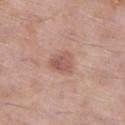– follow-up · catalogued during a skin exam; not biopsied
– automated metrics · an area of roughly 5.5 mm², an eccentricity of roughly 0.35, and a symmetry-axis asymmetry near 0.35; a mean CIELAB color near L≈55 a*≈22 b*≈25 and a lesion-to-skin contrast of about 6.5 (normalized; higher = more distinct); an automated nevus-likeness rating near 20 out of 100 and lesion-presence confidence of about 100/100
– lesion size · about 2.5 mm
– imaging modality · ~15 mm tile from a whole-body skin photo
– illumination · white-light
– patient · male, aged around 55
– body site · the left thigh